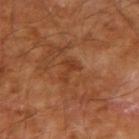Background:
The lesion's longest dimension is about 3 mm. The tile uses cross-polarized illumination. The lesion is on the right upper arm. A 15 mm crop from a total-body photograph taken for skin-cancer surveillance. A male patient, roughly 70 years of age. An algorithmic analysis of the crop reported a lesion–skin lightness drop of about 6 and a normalized border contrast of about 5.5.